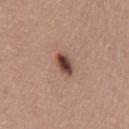  biopsy_status: not biopsied; imaged during a skin examination
  automated_metrics:
    area_mm2_approx: 4.5
    eccentricity: 0.8
    shape_asymmetry: 0.2
    nevus_likeness_0_100: 95
    lesion_detection_confidence_0_100: 100
  site: chest
  lighting: white-light
  patient:
    sex: male
    age_approx: 55
  lesion_size:
    long_diameter_mm_approx: 3.0
  image:
    source: total-body photography crop
    field_of_view_mm: 15